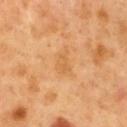Q: Is there a histopathology result?
A: catalogued during a skin exam; not biopsied
Q: Lesion location?
A: the back
Q: What are the patient's age and sex?
A: male, aged 48–52
Q: How large is the lesion?
A: ≈3 mm
Q: What did automated image analysis measure?
A: a lesion color around L≈62 a*≈23 b*≈45 in CIELAB, roughly 6 lightness units darker than nearby skin, and a normalized border contrast of about 5; a lesion-detection confidence of about 100/100
Q: How was the tile lit?
A: cross-polarized illumination
Q: What is the imaging modality?
A: 15 mm crop, total-body photography